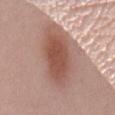Image and clinical context:
The lesion is located on the mid back. A female subject aged 58 to 62. Cropped from a total-body skin-imaging series; the visible field is about 15 mm. Longest diameter approximately 8 mm. Captured under white-light illumination.